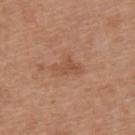| feature | finding |
|---|---|
| lighting | white-light |
| imaging modality | ~15 mm crop, total-body skin-cancer survey |
| anatomic site | the back |
| lesion size | ~3.5 mm (longest diameter) |
| subject | female, aged around 40 |